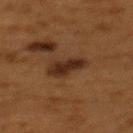No biopsy was performed on this lesion — it was imaged during a full skin examination and was not determined to be concerning.
A region of skin cropped from a whole-body photographic capture, roughly 15 mm wide.
The patient is a female about 50 years old.
The lesion's longest dimension is about 4.5 mm.
This is a cross-polarized tile.
On the upper back.
The total-body-photography lesion software estimated a lesion area of about 8.5 mm² and an eccentricity of roughly 0.85. It also reported a border-irregularity rating of about 3.5/10 and internal color variation of about 2.5 on a 0–10 scale.